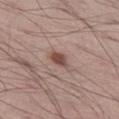patient: male, aged 68–72 | tile lighting: white-light illumination | image source: total-body-photography crop, ~15 mm field of view | image-analysis metrics: an area of roughly 3.5 mm², an outline eccentricity of about 0.8 (0 = round, 1 = elongated), and two-axis asymmetry of about 0.4; border irregularity of about 3.5 on a 0–10 scale, a color-variation rating of about 2/10, and radial color variation of about 0.5; a nevus-likeness score of about 90/100 and a detector confidence of about 100 out of 100 that the crop contains a lesion | site: the left thigh | lesion size: ~2.5 mm (longest diameter).